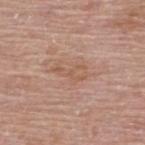Context:
Imaged with white-light lighting. This image is a 15 mm lesion crop taken from a total-body photograph. The lesion's longest dimension is about 4 mm. The lesion is located on the upper back. The subject is a male aged 78–82.